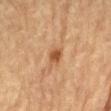Clinical summary: This image is a 15 mm lesion crop taken from a total-body photograph. About 2.5 mm across. Located on the abdomen. Captured under cross-polarized illumination. The patient is a male aged approximately 85 or older.